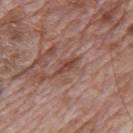Q: Was this lesion biopsied?
A: imaged on a skin check; not biopsied
Q: What did automated image analysis measure?
A: a border-irregularity rating of about 4.5/10, internal color variation of about 1.5 on a 0–10 scale, and peripheral color asymmetry of about 0.5
Q: Illumination type?
A: white-light illumination
Q: Who is the patient?
A: male, aged 68 to 72
Q: How large is the lesion?
A: ~3.5 mm (longest diameter)
Q: What kind of image is this?
A: total-body-photography crop, ~15 mm field of view
Q: Lesion location?
A: the mid back